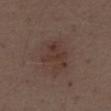Q: Was this lesion biopsied?
A: no biopsy performed (imaged during a skin exam)
Q: Who is the patient?
A: male, about 70 years old
Q: How was this image acquired?
A: total-body-photography crop, ~15 mm field of view
Q: What is the anatomic site?
A: the chest